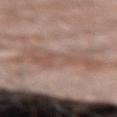Impression:
The lesion was tiled from a total-body skin photograph and was not biopsied.